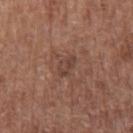Impression: No biopsy was performed on this lesion — it was imaged during a full skin examination and was not determined to be concerning. Acquisition and patient details: The tile uses white-light illumination. A male patient, about 65 years old. This image is a 15 mm lesion crop taken from a total-body photograph. The lesion's longest dimension is about 3 mm. The lesion is on the right upper arm. An algorithmic analysis of the crop reported a nevus-likeness score of about 0/100 and a lesion-detection confidence of about 95/100.